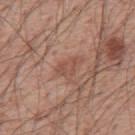site: mid back
image:
  source: total-body photography crop
  field_of_view_mm: 15
lighting: white-light
lesion_size:
  long_diameter_mm_approx: 3.0
patient:
  sex: male
  age_approx: 55